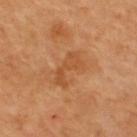Case summary:
- imaging modality: ~15 mm tile from a whole-body skin photo
- diameter: ~4.5 mm (longest diameter)
- image-analysis metrics: a lesion area of about 7.5 mm², an outline eccentricity of about 0.9 (0 = round, 1 = elongated), and a shape-asymmetry score of about 0.4 (0 = symmetric); a border-irregularity index near 6/10 and peripheral color asymmetry of about 0.5
- patient: male, aged around 45
- body site: the upper back
- lighting: cross-polarized